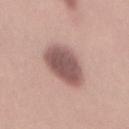Notes:
* workup · no biopsy performed (imaged during a skin exam)
* tile lighting · white-light
* image-analysis metrics · a footprint of about 17 mm², an outline eccentricity of about 0.8 (0 = round, 1 = elongated), and a symmetry-axis asymmetry near 0.15; an automated nevus-likeness rating near 50 out of 100 and a detector confidence of about 100 out of 100 that the crop contains a lesion
* lesion size · about 6 mm
* imaging modality · total-body-photography crop, ~15 mm field of view
* anatomic site · the mid back
* subject · female, aged 23 to 27